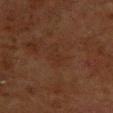A lesion tile, about 15 mm wide, cut from a 3D total-body photograph.
A female subject aged 78–82.
From the upper back.